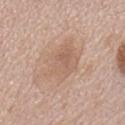Impression: Recorded during total-body skin imaging; not selected for excision or biopsy. Background: A 15 mm close-up extracted from a 3D total-body photography capture. A male subject, aged 68–72. The lesion is on the mid back. An algorithmic analysis of the crop reported a lesion color around L≈61 a*≈17 b*≈29 in CIELAB, roughly 7 lightness units darker than nearby skin, and a lesion-to-skin contrast of about 5 (normalized; higher = more distinct). It also reported an automated nevus-likeness rating near 0 out of 100 and a detector confidence of about 100 out of 100 that the crop contains a lesion. The tile uses white-light illumination. The recorded lesion diameter is about 5.5 mm.